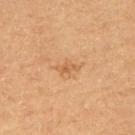| key | value |
|---|---|
| follow-up | catalogued during a skin exam; not biopsied |
| image source | ~15 mm crop, total-body skin-cancer survey |
| lighting | cross-polarized |
| subject | female, aged approximately 60 |
| location | the upper back |
| lesion size | ~3 mm (longest diameter) |